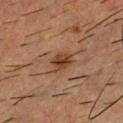Q: Was a biopsy performed?
A: imaged on a skin check; not biopsied
Q: How large is the lesion?
A: about 3 mm
Q: What kind of image is this?
A: ~15 mm tile from a whole-body skin photo
Q: How was the tile lit?
A: cross-polarized illumination
Q: What are the patient's age and sex?
A: male, aged 53 to 57
Q: What is the anatomic site?
A: the upper back